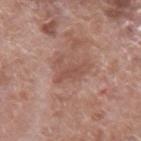Imaged during a routine full-body skin examination; the lesion was not biopsied and no histopathology is available. A male patient, approximately 60 years of age. The lesion's longest dimension is about 4 mm. The tile uses white-light illumination. The lesion is on the right upper arm. A lesion tile, about 15 mm wide, cut from a 3D total-body photograph. An algorithmic analysis of the crop reported an average lesion color of about L≈51 a*≈22 b*≈26 (CIELAB), roughly 8 lightness units darker than nearby skin, and a lesion-to-skin contrast of about 5.5 (normalized; higher = more distinct). It also reported a classifier nevus-likeness of about 0/100 and a detector confidence of about 100 out of 100 that the crop contains a lesion.